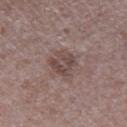<case>
<lighting>white-light</lighting>
<image>
  <source>total-body photography crop</source>
  <field_of_view_mm>15</field_of_view_mm>
</image>
<site>right lower leg</site>
<lesion_size>
  <long_diameter_mm_approx>3.5</long_diameter_mm_approx>
</lesion_size>
<patient>
  <sex>male</sex>
  <age_approx>45</age_approx>
</patient>
</case>